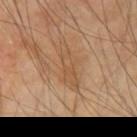No biopsy was performed on this lesion — it was imaged during a full skin examination and was not determined to be concerning. Located on the left upper arm. A male patient in their mid- to late 60s. A roughly 15 mm field-of-view crop from a total-body skin photograph.